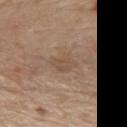Recorded during total-body skin imaging; not selected for excision or biopsy.
A male subject, aged approximately 70.
From the chest.
About 3 mm across.
A close-up tile cropped from a whole-body skin photograph, about 15 mm across.